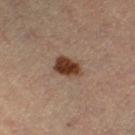patient:
  sex: female
  age_approx: 60
site: left thigh
image:
  source: total-body photography crop
  field_of_view_mm: 15